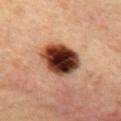Clinical summary: The patient is a female aged 38 to 42. Located on the mid back. A roughly 15 mm field-of-view crop from a total-body skin photograph. Automated tile analysis of the lesion measured an automated nevus-likeness rating near 95 out of 100 and a detector confidence of about 100 out of 100 that the crop contains a lesion. Approximately 5 mm at its widest. The tile uses cross-polarized illumination. Conclusion: The lesion was biopsied, and histopathology showed a compound melanocytic nevus.